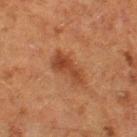workup: catalogued during a skin exam; not biopsied
subject: female, in their 50s
image: ~15 mm crop, total-body skin-cancer survey
anatomic site: the left thigh
lighting: cross-polarized
lesion diameter: ~4.5 mm (longest diameter)